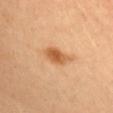- patient: female, aged 33–37
- acquisition: 15 mm crop, total-body photography
- anatomic site: the head or neck
- lighting: cross-polarized illumination
- size: about 3.5 mm